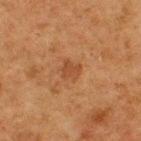Captured during whole-body skin photography for melanoma surveillance; the lesion was not biopsied.
A male subject, in their mid-70s.
Located on the upper back.
A 15 mm close-up tile from a total-body photography series done for melanoma screening.